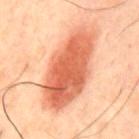workup — no biopsy performed (imaged during a skin exam)